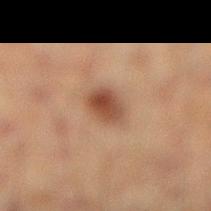Notes:
* workup · imaged on a skin check; not biopsied
* tile lighting · cross-polarized illumination
* site · the right lower leg
* TBP lesion metrics · an average lesion color of about L≈37 a*≈17 b*≈25 (CIELAB) and about 10 CIELAB-L* units darker than the surrounding skin; a classifier nevus-likeness of about 95/100 and lesion-presence confidence of about 100/100
* lesion diameter · about 3.5 mm
* patient · male, roughly 50 years of age
* image source · total-body-photography crop, ~15 mm field of view